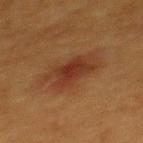<case>
  <biopsy_status>not biopsied; imaged during a skin examination</biopsy_status>
  <image>
    <source>total-body photography crop</source>
    <field_of_view_mm>15</field_of_view_mm>
  </image>
  <lesion_size>
    <long_diameter_mm_approx>6.0</long_diameter_mm_approx>
  </lesion_size>
  <patient>
    <sex>female</sex>
    <age_approx>40</age_approx>
  </patient>
  <site>upper back</site>
  <lighting>cross-polarized</lighting>
</case>